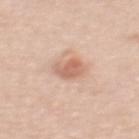Clinical impression: Imaged during a routine full-body skin examination; the lesion was not biopsied and no histopathology is available. Context: Longest diameter approximately 2.5 mm. An algorithmic analysis of the crop reported a footprint of about 5.5 mm² and an outline eccentricity of about 0.35 (0 = round, 1 = elongated). The analysis additionally found a lesion color around L≈63 a*≈23 b*≈30 in CIELAB, a lesion–skin lightness drop of about 11, and a normalized border contrast of about 7. It also reported a border-irregularity index near 2.5/10 and a peripheral color-asymmetry measure near 1. Captured under white-light illumination. Located on the mid back. The subject is a female aged 43–47. This image is a 15 mm lesion crop taken from a total-body photograph.